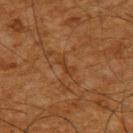workup — catalogued during a skin exam; not biopsied | tile lighting — cross-polarized illumination | automated metrics — a shape eccentricity near 0.9 and a shape-asymmetry score of about 0.4 (0 = symmetric) | subject — male, aged approximately 65 | lesion size — about 2.5 mm | imaging modality — 15 mm crop, total-body photography | site — the upper back.